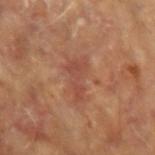| feature | finding |
|---|---|
| follow-up | no biopsy performed (imaged during a skin exam) |
| tile lighting | cross-polarized illumination |
| patient | male, roughly 65 years of age |
| body site | the left forearm |
| imaging modality | ~15 mm tile from a whole-body skin photo |